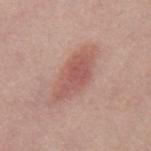The patient is a male aged 38 to 42.
Cropped from a whole-body photographic skin survey; the tile spans about 15 mm.
Measured at roughly 6.5 mm in maximum diameter.
This is a white-light tile.
The lesion is on the mid back.